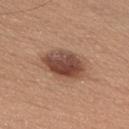Captured during whole-body skin photography for melanoma surveillance; the lesion was not biopsied. This is a white-light tile. Automated image analysis of the tile measured a mean CIELAB color near L≈45 a*≈22 b*≈27 and a normalized border contrast of about 11. And it measured a border-irregularity rating of about 2/10, a within-lesion color-variation index near 6.5/10, and radial color variation of about 2. The analysis additionally found an automated nevus-likeness rating near 90 out of 100 and lesion-presence confidence of about 100/100. A roughly 15 mm field-of-view crop from a total-body skin photograph. The lesion is located on the chest. A male patient aged approximately 30. The recorded lesion diameter is about 5 mm.